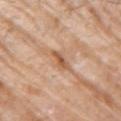  biopsy_status: not biopsied; imaged during a skin examination
  site: right upper arm
  lighting: white-light
  image:
    source: total-body photography crop
    field_of_view_mm: 15
  automated_metrics:
    area_mm2_approx: 4.0
    shape_asymmetry: 0.4
    cielab_L: 58
    cielab_a: 21
    cielab_b: 35
    vs_skin_darker_L: 11.0
    vs_skin_contrast_norm: 7.5
    nevus_likeness_0_100: 0
    lesion_detection_confidence_0_100: 85
  lesion_size:
    long_diameter_mm_approx: 3.5
  patient:
    sex: male
    age_approx: 80